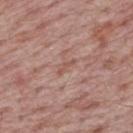follow-up = imaged on a skin check; not biopsied
image = 15 mm crop, total-body photography
automated metrics = a lesion area of about 2 mm², an eccentricity of roughly 0.95, and a symmetry-axis asymmetry near 0.6; an average lesion color of about L≈53 a*≈22 b*≈26 (CIELAB) and a lesion–skin lightness drop of about 7
anatomic site = the upper back
subject = male, roughly 65 years of age